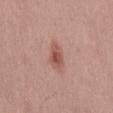Context: Automated image analysis of the tile measured a footprint of about 4.5 mm², a shape eccentricity near 0.85, and a shape-asymmetry score of about 0.35 (0 = symmetric). It also reported a mean CIELAB color near L≈52 a*≈24 b*≈25, roughly 11 lightness units darker than nearby skin, and a lesion-to-skin contrast of about 7.5 (normalized; higher = more distinct). And it measured border irregularity of about 3 on a 0–10 scale and peripheral color asymmetry of about 1. It also reported a nevus-likeness score of about 75/100. From the back. Longest diameter approximately 3 mm. Imaged with white-light lighting. A male subject, aged 43–47. A roughly 15 mm field-of-view crop from a total-body skin photograph.